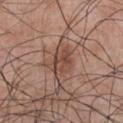Assessment:
No biopsy was performed on this lesion — it was imaged during a full skin examination and was not determined to be concerning.
Background:
Measured at roughly 4 mm in maximum diameter. The tile uses white-light illumination. Located on the chest. The total-body-photography lesion software estimated a mean CIELAB color near L≈46 a*≈20 b*≈26, a lesion–skin lightness drop of about 9, and a normalized lesion–skin contrast near 7.5. The analysis additionally found peripheral color asymmetry of about 2. The software also gave a classifier nevus-likeness of about 15/100 and a detector confidence of about 100 out of 100 that the crop contains a lesion. The subject is a male approximately 70 years of age. A roughly 15 mm field-of-view crop from a total-body skin photograph.